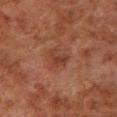notes = total-body-photography surveillance lesion; no biopsy | tile lighting = cross-polarized illumination | location = the left lower leg | image = ~15 mm crop, total-body skin-cancer survey | subject = male, aged approximately 80.